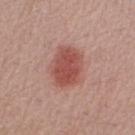Clinical impression:
Part of a total-body skin-imaging series; this lesion was reviewed on a skin check and was not flagged for biopsy.
Acquisition and patient details:
This is a white-light tile. A close-up tile cropped from a whole-body skin photograph, about 15 mm across. On the right forearm. The lesion's longest dimension is about 5 mm. A female subject aged approximately 50.